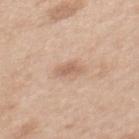notes: catalogued during a skin exam; not biopsied | image source: total-body-photography crop, ~15 mm field of view | patient: female, aged around 40 | site: the mid back | TBP lesion metrics: an area of roughly 3.5 mm², a shape eccentricity near 0.75, and two-axis asymmetry of about 0.25; lesion-presence confidence of about 100/100.